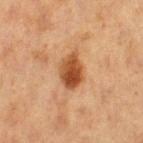No biopsy was performed on this lesion — it was imaged during a full skin examination and was not determined to be concerning.
Captured under cross-polarized illumination.
This image is a 15 mm lesion crop taken from a total-body photograph.
A female patient, approximately 40 years of age.
An algorithmic analysis of the crop reported a color-variation rating of about 6/10 and radial color variation of about 2. The analysis additionally found a nevus-likeness score of about 100/100 and a lesion-detection confidence of about 100/100.
The lesion is on the left thigh.
Approximately 4 mm at its widest.